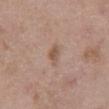Q: Was this lesion biopsied?
A: imaged on a skin check; not biopsied
Q: Lesion size?
A: ≈2.5 mm
Q: What kind of image is this?
A: 15 mm crop, total-body photography
Q: Where on the body is the lesion?
A: the abdomen
Q: Who is the patient?
A: male, about 50 years old